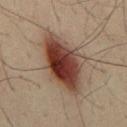Clinical impression: The lesion was photographed on a routine skin check and not biopsied; there is no pathology result. Acquisition and patient details: Imaged with cross-polarized lighting. A male patient aged 48 to 52. Cropped from a total-body skin-imaging series; the visible field is about 15 mm. Longest diameter approximately 9 mm. The lesion is on the mid back.